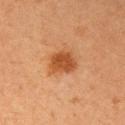Captured during whole-body skin photography for melanoma surveillance; the lesion was not biopsied.
A female subject, aged 38–42.
Approximately 3.5 mm at its widest.
The lesion is on the right upper arm.
The tile uses cross-polarized illumination.
Automated tile analysis of the lesion measured a classifier nevus-likeness of about 100/100 and a detector confidence of about 100 out of 100 that the crop contains a lesion.
A 15 mm crop from a total-body photograph taken for skin-cancer surveillance.